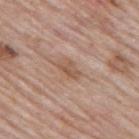This lesion was catalogued during total-body skin photography and was not selected for biopsy. Automated tile analysis of the lesion measured an area of roughly 4 mm² and an eccentricity of roughly 0.8. A male subject, roughly 60 years of age. From the upper back. Imaged with white-light lighting. Longest diameter approximately 3 mm. A 15 mm close-up extracted from a 3D total-body photography capture.